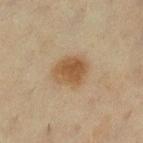anatomic site = the left lower leg | diameter = ≈4 mm | lighting = cross-polarized | patient = female, aged 53 to 57 | image source = 15 mm crop, total-body photography.